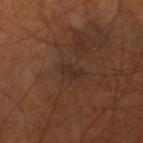<tbp_lesion>
  <biopsy_status>not biopsied; imaged during a skin examination</biopsy_status>
  <image>
    <source>total-body photography crop</source>
    <field_of_view_mm>15</field_of_view_mm>
  </image>
  <site>leg</site>
  <patient>
    <sex>male</sex>
    <age_approx>70</age_approx>
  </patient>
  <automated_metrics>
    <area_mm2_approx>3.5</area_mm2_approx>
    <eccentricity>0.9</eccentricity>
    <shape_asymmetry>0.35</shape_asymmetry>
    <cielab_L>27</cielab_L>
    <cielab_a>16</cielab_a>
    <cielab_b>21</cielab_b>
    <vs_skin_darker_L>5.0</vs_skin_darker_L>
    <vs_skin_contrast_norm>7.0</vs_skin_contrast_norm>
    <color_variation_0_10>0.5</color_variation_0_10>
  </automated_metrics>
  <lighting>cross-polarized</lighting>
</tbp_lesion>